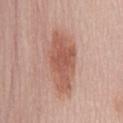Findings:
- biopsy status: total-body-photography surveillance lesion; no biopsy
- subject: female, aged approximately 55
- anatomic site: the mid back
- tile lighting: white-light illumination
- imaging modality: ~15 mm crop, total-body skin-cancer survey
- lesion diameter: ≈8 mm